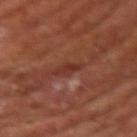No biopsy was performed on this lesion — it was imaged during a full skin examination and was not determined to be concerning.
A male subject, about 65 years old.
Cropped from a total-body skin-imaging series; the visible field is about 15 mm.
The tile uses cross-polarized illumination.
Automated image analysis of the tile measured a border-irregularity rating of about 3/10 and a peripheral color-asymmetry measure near 0.5.
Located on the arm.